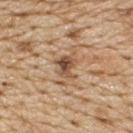workup = catalogued during a skin exam; not biopsied | automated metrics = a border-irregularity rating of about 4.5/10 and a peripheral color-asymmetry measure near 2.5 | tile lighting = white-light | acquisition = 15 mm crop, total-body photography | lesion size = about 3 mm | location = the upper back | subject = male, aged around 70.